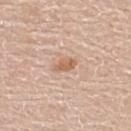The lesion is located on the back.
A lesion tile, about 15 mm wide, cut from a 3D total-body photograph.
Automated tile analysis of the lesion measured a nevus-likeness score of about 30/100 and a detector confidence of about 100 out of 100 that the crop contains a lesion.
A male patient aged approximately 60.